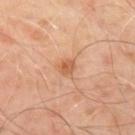Part of a total-body skin-imaging series; this lesion was reviewed on a skin check and was not flagged for biopsy.
This image is a 15 mm lesion crop taken from a total-body photograph.
About 2 mm across.
On the upper back.
A male patient in their 50s.
The tile uses cross-polarized illumination.